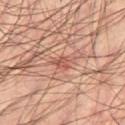Part of a total-body skin-imaging series; this lesion was reviewed on a skin check and was not flagged for biopsy. A 15 mm close-up tile from a total-body photography series done for melanoma screening. An algorithmic analysis of the crop reported a border-irregularity rating of about 3.5/10, internal color variation of about 2 on a 0–10 scale, and peripheral color asymmetry of about 1. The software also gave an automated nevus-likeness rating near 0 out of 100. Located on the right thigh. The patient is a male approximately 60 years of age. Imaged with cross-polarized lighting. Approximately 2.5 mm at its widest.